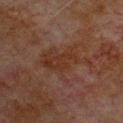Notes:
– follow-up — no biopsy performed (imaged during a skin exam)
– image source — 15 mm crop, total-body photography
– site — the front of the torso
– TBP lesion metrics — a footprint of about 15 mm², an eccentricity of roughly 0.75, and two-axis asymmetry of about 0.45; roughly 4 lightness units darker than nearby skin; a classifier nevus-likeness of about 0/100 and lesion-presence confidence of about 100/100
– illumination — cross-polarized
– patient — male, aged around 80